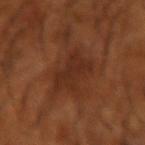Clinical impression: Captured during whole-body skin photography for melanoma surveillance; the lesion was not biopsied. Context: A male subject in their mid- to late 60s. A 15 mm close-up tile from a total-body photography series done for melanoma screening. On the right forearm. An algorithmic analysis of the crop reported border irregularity of about 4 on a 0–10 scale, internal color variation of about 2 on a 0–10 scale, and radial color variation of about 0.5. The analysis additionally found a lesion-detection confidence of about 90/100. The recorded lesion diameter is about 5 mm.